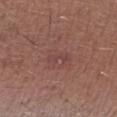follow-up = catalogued during a skin exam; not biopsied | subject = male, aged 63–67 | anatomic site = the left lower leg | imaging modality = 15 mm crop, total-body photography.